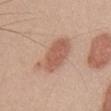Recorded during total-body skin imaging; not selected for excision or biopsy.
The subject is a male in their 50s.
The lesion's longest dimension is about 5.5 mm.
A 15 mm crop from a total-body photograph taken for skin-cancer surveillance.
Located on the front of the torso.
The tile uses white-light illumination.